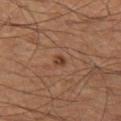The lesion was photographed on a routine skin check and not biopsied; there is no pathology result. The patient is a male roughly 60 years of age. From the left thigh. A 15 mm close-up tile from a total-body photography series done for melanoma screening. The tile uses cross-polarized illumination.